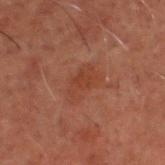Q: Was this lesion biopsied?
A: no biopsy performed (imaged during a skin exam)
Q: What is the imaging modality?
A: 15 mm crop, total-body photography
Q: Lesion size?
A: about 4 mm
Q: What is the anatomic site?
A: the head or neck
Q: What did automated image analysis measure?
A: an area of roughly 7.5 mm²; a border-irregularity rating of about 4/10, a within-lesion color-variation index near 1.5/10, and a peripheral color-asymmetry measure near 0.5
Q: What are the patient's age and sex?
A: male, in their 60s